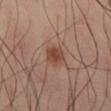Assessment: Captured during whole-body skin photography for melanoma surveillance; the lesion was not biopsied. Context: Automated image analysis of the tile measured border irregularity of about 2 on a 0–10 scale, a color-variation rating of about 3/10, and a peripheral color-asymmetry measure near 1. And it measured a nevus-likeness score of about 95/100 and lesion-presence confidence of about 100/100. Cropped from a whole-body photographic skin survey; the tile spans about 15 mm. The lesion's longest dimension is about 3 mm. Located on the right thigh. This is a cross-polarized tile. A male subject, aged approximately 40.